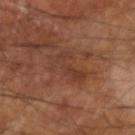The lesion was tiled from a total-body skin photograph and was not biopsied. On the left arm. A 15 mm crop from a total-body photograph taken for skin-cancer surveillance. A male subject aged 63–67. Imaged with cross-polarized lighting.